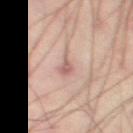A male patient, about 40 years old.
Imaged with cross-polarized lighting.
A lesion tile, about 15 mm wide, cut from a 3D total-body photograph.
From the left thigh.
The lesion's longest dimension is about 3 mm.
An algorithmic analysis of the crop reported a classifier nevus-likeness of about 0/100.